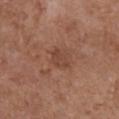Imaged during a routine full-body skin examination; the lesion was not biopsied and no histopathology is available.
On the front of the torso.
A 15 mm close-up tile from a total-body photography series done for melanoma screening.
The recorded lesion diameter is about 3 mm.
The tile uses white-light illumination.
A female subject roughly 80 years of age.